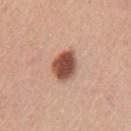Part of a total-body skin-imaging series; this lesion was reviewed on a skin check and was not flagged for biopsy. The lesion's longest dimension is about 3.5 mm. This is a white-light tile. Located on the left upper arm. A female patient, aged 38 to 42. A roughly 15 mm field-of-view crop from a total-body skin photograph.